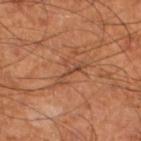Assessment: This lesion was catalogued during total-body skin photography and was not selected for biopsy. Context: A lesion tile, about 15 mm wide, cut from a 3D total-body photograph. From the left lower leg. Captured under cross-polarized illumination. The lesion-visualizer software estimated border irregularity of about 9.5 on a 0–10 scale and a within-lesion color-variation index near 0/10. A male subject approximately 60 years of age. About 4 mm across.